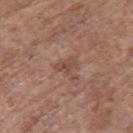Part of a total-body skin-imaging series; this lesion was reviewed on a skin check and was not flagged for biopsy. A female patient aged 63–67. A lesion tile, about 15 mm wide, cut from a 3D total-body photograph. Captured under white-light illumination. Longest diameter approximately 2.5 mm. The lesion is on the upper back.